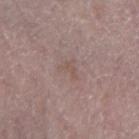Q: Was this lesion biopsied?
A: no biopsy performed (imaged during a skin exam)
Q: How was this image acquired?
A: ~15 mm crop, total-body skin-cancer survey
Q: What is the anatomic site?
A: the right forearm
Q: What are the patient's age and sex?
A: female, aged 63–67
Q: What lighting was used for the tile?
A: white-light illumination